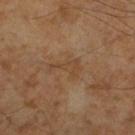The patient is a male aged around 60. The lesion is on the left lower leg. About 4.5 mm across. A 15 mm close-up extracted from a 3D total-body photography capture. The tile uses cross-polarized illumination.